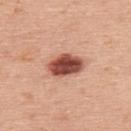This lesion was catalogued during total-body skin photography and was not selected for biopsy.
The lesion is located on the upper back.
This image is a 15 mm lesion crop taken from a total-body photograph.
A male patient, aged 73 to 77.